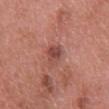workup: imaged on a skin check; not biopsied
subject: female, roughly 70 years of age
anatomic site: the front of the torso
tile lighting: white-light illumination
TBP lesion metrics: an area of roughly 4.5 mm²; internal color variation of about 3.5 on a 0–10 scale and radial color variation of about 1; a nevus-likeness score of about 30/100 and lesion-presence confidence of about 100/100
imaging modality: 15 mm crop, total-body photography